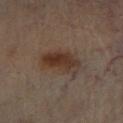<case>
  <biopsy_status>not biopsied; imaged during a skin examination</biopsy_status>
  <patient>
    <sex>male</sex>
    <age_approx>70</age_approx>
  </patient>
  <lighting>cross-polarized</lighting>
  <image>
    <source>total-body photography crop</source>
    <field_of_view_mm>15</field_of_view_mm>
  </image>
  <lesion_size>
    <long_diameter_mm_approx>5.0</long_diameter_mm_approx>
  </lesion_size>
  <site>right lower leg</site>
</case>